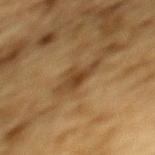| feature | finding |
|---|---|
| workup | total-body-photography surveillance lesion; no biopsy |
| diameter | ~4.5 mm (longest diameter) |
| imaging modality | 15 mm crop, total-body photography |
| subject | male, aged 83 or older |
| anatomic site | the mid back |